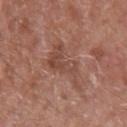The lesion was photographed on a routine skin check and not biopsied; there is no pathology result.